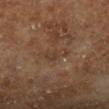The lesion was tiled from a total-body skin photograph and was not biopsied.
The patient is a male aged 63 to 67.
The lesion's longest dimension is about 3.5 mm.
Captured under cross-polarized illumination.
A region of skin cropped from a whole-body photographic capture, roughly 15 mm wide.